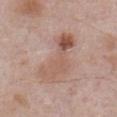follow-up: imaged on a skin check; not biopsied | size: ≈7 mm | automated lesion analysis: a lesion area of about 16 mm², a shape eccentricity near 0.9, and a shape-asymmetry score of about 0.45 (0 = symmetric); a lesion color around L≈56 a*≈19 b*≈27 in CIELAB, roughly 9 lightness units darker than nearby skin, and a lesion-to-skin contrast of about 6.5 (normalized; higher = more distinct); a border-irregularity rating of about 6.5/10, a within-lesion color-variation index near 9/10, and a peripheral color-asymmetry measure near 3 | patient: male, aged 53 to 57 | body site: the abdomen | imaging modality: ~15 mm crop, total-body skin-cancer survey.